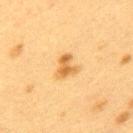biopsy_status: not biopsied; imaged during a skin examination
patient:
  sex: female
  age_approx: 40
image:
  source: total-body photography crop
  field_of_view_mm: 15
site: upper back
lesion_size:
  long_diameter_mm_approx: 3.0